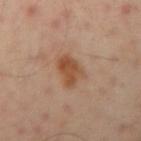Part of a total-body skin-imaging series; this lesion was reviewed on a skin check and was not flagged for biopsy. A male subject, aged 48–52. The recorded lesion diameter is about 3.5 mm. Captured under cross-polarized illumination. From the left arm. Cropped from a whole-body photographic skin survey; the tile spans about 15 mm. The lesion-visualizer software estimated a lesion area of about 7.5 mm², an outline eccentricity of about 0.65 (0 = round, 1 = elongated), and a shape-asymmetry score of about 0.3 (0 = symmetric). The software also gave about 9 CIELAB-L* units darker than the surrounding skin and a normalized border contrast of about 8.5. The analysis additionally found border irregularity of about 3 on a 0–10 scale, internal color variation of about 3 on a 0–10 scale, and peripheral color asymmetry of about 1.